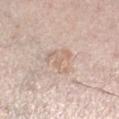Imaged during a routine full-body skin examination; the lesion was not biopsied and no histopathology is available.